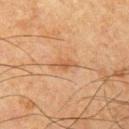The lesion was tiled from a total-body skin photograph and was not biopsied.
Cropped from a whole-body photographic skin survey; the tile spans about 15 mm.
The tile uses cross-polarized illumination.
About 3 mm across.
The total-body-photography lesion software estimated a shape-asymmetry score of about 0.3 (0 = symmetric). The software also gave a lesion-detection confidence of about 100/100.
The patient is a male aged 63–67.
The lesion is on the left upper arm.